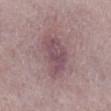Assessment: The lesion was tiled from a total-body skin photograph and was not biopsied. Background: Automated image analysis of the tile measured an area of roughly 16 mm² and two-axis asymmetry of about 0.25. This image is a 15 mm lesion crop taken from a total-body photograph. This is a white-light tile. A female subject roughly 60 years of age. The lesion is located on the right lower leg.